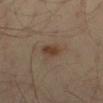<lesion>
<patient>
  <sex>male</sex>
  <age_approx>35</age_approx>
</patient>
<image>
  <source>total-body photography crop</source>
  <field_of_view_mm>15</field_of_view_mm>
</image>
<site>leg</site>
<lesion_size>
  <long_diameter_mm_approx>3.0</long_diameter_mm_approx>
</lesion_size>
</lesion>